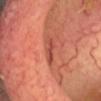Q: Was this lesion biopsied?
A: imaged on a skin check; not biopsied
Q: Patient demographics?
A: male, aged around 60
Q: Automated lesion metrics?
A: a border-irregularity rating of about 4/10 and a peripheral color-asymmetry measure near 0.5; a nevus-likeness score of about 0/100 and a lesion-detection confidence of about 55/100
Q: Lesion size?
A: about 3.5 mm
Q: What kind of image is this?
A: total-body-photography crop, ~15 mm field of view
Q: Lesion location?
A: the head or neck